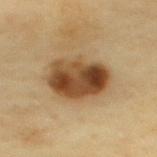Cropped from a whole-body photographic skin survey; the tile spans about 15 mm. The lesion's longest dimension is about 6.5 mm. Captured under cross-polarized illumination. A female subject about 60 years old. An algorithmic analysis of the crop reported a footprint of about 23 mm² and an outline eccentricity of about 0.65 (0 = round, 1 = elongated). And it measured a mean CIELAB color near L≈43 a*≈17 b*≈33, a lesion–skin lightness drop of about 15, and a lesion-to-skin contrast of about 11.5 (normalized; higher = more distinct). The lesion is located on the upper back.